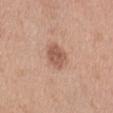biopsy_status: not biopsied; imaged during a skin examination
lighting: white-light
image:
  source: total-body photography crop
  field_of_view_mm: 15
patient:
  sex: female
  age_approx: 40
lesion_size:
  long_diameter_mm_approx: 3.5
site: left thigh